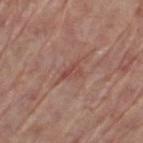The lesion was tiled from a total-body skin photograph and was not biopsied. Cropped from a whole-body photographic skin survey; the tile spans about 15 mm. Automated tile analysis of the lesion measured a mean CIELAB color near L≈48 a*≈23 b*≈25 and a normalized border contrast of about 5. It also reported a border-irregularity index near 4/10, internal color variation of about 3 on a 0–10 scale, and radial color variation of about 1. Located on the left thigh. This is a white-light tile. The recorded lesion diameter is about 3 mm. A male patient about 70 years old.